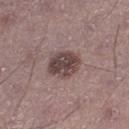This lesion was catalogued during total-body skin photography and was not selected for biopsy.
A 15 mm close-up tile from a total-body photography series done for melanoma screening.
Located on the right lower leg.
A male patient in their mid-40s.
Approximately 4 mm at its widest.